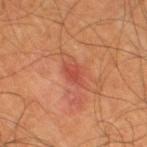follow-up = total-body-photography surveillance lesion; no biopsy | image = 15 mm crop, total-body photography | site = the leg | subject = male, roughly 65 years of age | TBP lesion metrics = an average lesion color of about L≈45 a*≈32 b*≈31 (CIELAB) and a lesion–skin lightness drop of about 8; a border-irregularity index near 3/10, a within-lesion color-variation index near 1.5/10, and a peripheral color-asymmetry measure near 0.5; an automated nevus-likeness rating near 10 out of 100 and lesion-presence confidence of about 100/100.